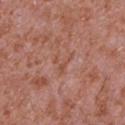{"biopsy_status": "not biopsied; imaged during a skin examination", "site": "chest", "automated_metrics": {"eccentricity": 0.7, "shape_asymmetry": 0.8, "lesion_detection_confidence_0_100": 90}, "patient": {"sex": "male", "age_approx": 45}, "lighting": "white-light", "image": {"source": "total-body photography crop", "field_of_view_mm": 15}, "lesion_size": {"long_diameter_mm_approx": 3.0}}